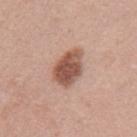From the left upper arm.
A female subject, in their mid-30s.
This image is a 15 mm lesion crop taken from a total-body photograph.
Captured under white-light illumination.